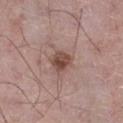| key | value |
|---|---|
| notes | catalogued during a skin exam; not biopsied |
| lighting | white-light illumination |
| diameter | ~3 mm (longest diameter) |
| patient | male, about 50 years old |
| imaging modality | ~15 mm tile from a whole-body skin photo |
| anatomic site | the left lower leg |